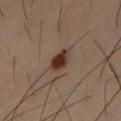{
  "biopsy_status": "not biopsied; imaged during a skin examination",
  "image": {
    "source": "total-body photography crop",
    "field_of_view_mm": 15
  },
  "lesion_size": {
    "long_diameter_mm_approx": 3.0
  },
  "site": "left thigh",
  "lighting": "cross-polarized",
  "patient": {
    "sex": "male",
    "age_approx": 30
  },
  "automated_metrics": {
    "cielab_L": 26,
    "cielab_a": 15,
    "cielab_b": 20,
    "vs_skin_darker_L": 12.0,
    "vs_skin_contrast_norm": 12.0,
    "nevus_likeness_0_100": 100,
    "lesion_detection_confidence_0_100": 100
  }
}